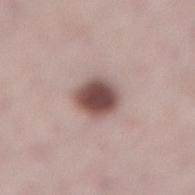Recorded during total-body skin imaging; not selected for excision or biopsy.
A female subject, aged 38 to 42.
The lesion-visualizer software estimated about 18 CIELAB-L* units darker than the surrounding skin. The analysis additionally found a border-irregularity index near 1.5/10, a within-lesion color-variation index near 4.5/10, and radial color variation of about 1. And it measured a classifier nevus-likeness of about 95/100.
The lesion's longest dimension is about 3.5 mm.
The lesion is located on the lower back.
A 15 mm crop from a total-body photograph taken for skin-cancer surveillance.